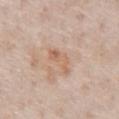| feature | finding |
|---|---|
| workup | no biopsy performed (imaged during a skin exam) |
| image | ~15 mm crop, total-body skin-cancer survey |
| location | the chest |
| subject | male, in their mid-70s |
| size | ~4 mm (longest diameter) |
| tile lighting | white-light illumination |